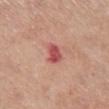follow-up = total-body-photography surveillance lesion; no biopsy | body site = the right lower leg | image source = ~15 mm tile from a whole-body skin photo | tile lighting = white-light | subject = female, roughly 45 years of age.